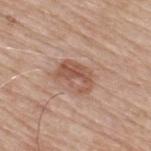biopsy_status: not biopsied; imaged during a skin examination
lighting: white-light
lesion_size:
  long_diameter_mm_approx: 4.0
patient:
  sex: male
  age_approx: 65
image:
  source: total-body photography crop
  field_of_view_mm: 15
site: back